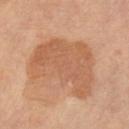| key | value |
|---|---|
| follow-up | catalogued during a skin exam; not biopsied |
| lesion diameter | ≈8.5 mm |
| body site | the leg |
| subject | female, aged approximately 65 |
| tile lighting | cross-polarized |
| TBP lesion metrics | a border-irregularity index near 4.5/10, a within-lesion color-variation index near 3/10, and peripheral color asymmetry of about 1 |
| imaging modality | ~15 mm crop, total-body skin-cancer survey |